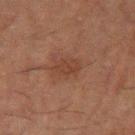biopsy_status: not biopsied; imaged during a skin examination